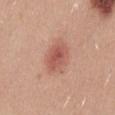<case>
<biopsy_status>not biopsied; imaged during a skin examination</biopsy_status>
<automated_metrics>
  <area_mm2_approx>10.0</area_mm2_approx>
  <shape_asymmetry>0.15</shape_asymmetry>
  <border_irregularity_0_10>1.5</border_irregularity_0_10>
  <color_variation_0_10>4.0</color_variation_0_10>
  <peripheral_color_asymmetry>1.0</peripheral_color_asymmetry>
  <nevus_likeness_0_100>95</nevus_likeness_0_100>
</automated_metrics>
<lighting>white-light</lighting>
<lesion_size>
  <long_diameter_mm_approx>4.0</long_diameter_mm_approx>
</lesion_size>
<image>
  <source>total-body photography crop</source>
  <field_of_view_mm>15</field_of_view_mm>
</image>
<patient>
  <sex>male</sex>
  <age_approx>25</age_approx>
</patient>
<site>mid back</site>
</case>